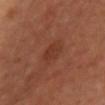Impression:
Imaged during a routine full-body skin examination; the lesion was not biopsied and no histopathology is available.
Acquisition and patient details:
A female patient, aged around 40. The lesion is on the front of the torso. Longest diameter approximately 2.5 mm. This is a cross-polarized tile. Cropped from a whole-body photographic skin survey; the tile spans about 15 mm.